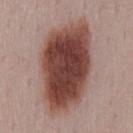Findings:
– biopsy status: no biopsy performed (imaged during a skin exam)
– illumination: white-light illumination
– TBP lesion metrics: a border-irregularity rating of about 2/10, a within-lesion color-variation index near 7.5/10, and radial color variation of about 2.5
– acquisition: ~15 mm tile from a whole-body skin photo
– location: the mid back
– subject: male, about 50 years old